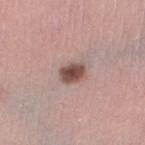<case>
  <biopsy_status>not biopsied; imaged during a skin examination</biopsy_status>
  <patient>
    <sex>female</sex>
    <age_approx>35</age_approx>
  </patient>
  <lesion_size>
    <long_diameter_mm_approx>3.0</long_diameter_mm_approx>
  </lesion_size>
  <image>
    <source>total-body photography crop</source>
    <field_of_view_mm>15</field_of_view_mm>
  </image>
  <automated_metrics>
    <cielab_L>50</cielab_L>
    <cielab_a>18</cielab_a>
    <cielab_b>22</cielab_b>
    <vs_skin_darker_L>15.0</vs_skin_darker_L>
    <vs_skin_contrast_norm>10.5</vs_skin_contrast_norm>
    <nevus_likeness_0_100>85</nevus_likeness_0_100>
    <lesion_detection_confidence_0_100>100</lesion_detection_confidence_0_100>
  </automated_metrics>
  <lighting>white-light</lighting>
  <site>left thigh</site>
</case>